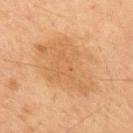{
  "automated_metrics": {
    "area_mm2_approx": 35.0,
    "eccentricity": 0.7,
    "shape_asymmetry": 0.25,
    "cielab_L": 55,
    "cielab_a": 19,
    "cielab_b": 36,
    "vs_skin_darker_L": 6.0,
    "vs_skin_contrast_norm": 5.0,
    "nevus_likeness_0_100": 0
  },
  "patient": {
    "sex": "male",
    "age_approx": 60
  },
  "lesion_size": {
    "long_diameter_mm_approx": 8.5
  },
  "lighting": "cross-polarized",
  "image": {
    "source": "total-body photography crop",
    "field_of_view_mm": 15
  }
}